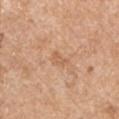{"biopsy_status": "not biopsied; imaged during a skin examination", "lighting": "white-light", "image": {"source": "total-body photography crop", "field_of_view_mm": 15}, "site": "left upper arm", "lesion_size": {"long_diameter_mm_approx": 2.5}, "patient": {"sex": "male", "age_approx": 55}}